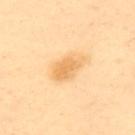biopsy_status: not biopsied; imaged during a skin examination
lighting: cross-polarized
lesion_size:
  long_diameter_mm_approx: 4.5
automated_metrics:
  cielab_L: 76
  cielab_a: 19
  cielab_b: 47
  vs_skin_contrast_norm: 6.5
  lesion_detection_confidence_0_100: 100
site: upper back
patient:
  sex: female
  age_approx: 40
image:
  source: total-body photography crop
  field_of_view_mm: 15